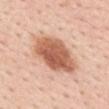Q: How was the tile lit?
A: white-light illumination
Q: What is the anatomic site?
A: the mid back
Q: What is the lesion's diameter?
A: about 6.5 mm
Q: How was this image acquired?
A: ~15 mm tile from a whole-body skin photo
Q: What are the patient's age and sex?
A: female, aged around 45
Q: What did automated image analysis measure?
A: a footprint of about 21 mm² and an outline eccentricity of about 0.75 (0 = round, 1 = elongated); an average lesion color of about L≈60 a*≈24 b*≈32 (CIELAB), a lesion–skin lightness drop of about 16, and a lesion-to-skin contrast of about 10 (normalized; higher = more distinct); a border-irregularity index near 2/10, a color-variation rating of about 5/10, and a peripheral color-asymmetry measure near 1.5